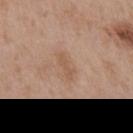follow-up — imaged on a skin check; not biopsied | patient — male, in their mid-60s | anatomic site — the chest | image source — 15 mm crop, total-body photography.